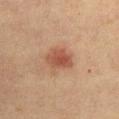biopsy_status: not biopsied; imaged during a skin examination
lesion_size:
  long_diameter_mm_approx: 3.5
site: abdomen
lighting: cross-polarized
patient:
  sex: female
  age_approx: 40
image:
  source: total-body photography crop
  field_of_view_mm: 15
automated_metrics:
  shape_asymmetry: 0.2
  border_irregularity_0_10: 2.0
  color_variation_0_10: 3.5
  peripheral_color_asymmetry: 1.0
  lesion_detection_confidence_0_100: 100